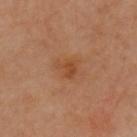biopsy status: no biopsy performed (imaged during a skin exam) | tile lighting: cross-polarized illumination | patient: female, approximately 60 years of age | site: the chest | lesion diameter: about 3 mm | acquisition: ~15 mm crop, total-body skin-cancer survey.